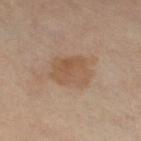No biopsy was performed on this lesion — it was imaged during a full skin examination and was not determined to be concerning. Captured under cross-polarized illumination. The patient is a female aged 48–52. A 15 mm crop from a total-body photograph taken for skin-cancer surveillance. From the right thigh. The lesion's longest dimension is about 4.5 mm.